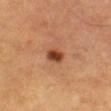follow-up — imaged on a skin check; not biopsied | subject — male, aged approximately 60 | automated lesion analysis — a lesion area of about 4.5 mm², a shape eccentricity near 0.6, and two-axis asymmetry of about 0.2; a border-irregularity rating of about 1.5/10, internal color variation of about 4 on a 0–10 scale, and radial color variation of about 1; an automated nevus-likeness rating near 100 out of 100 and a lesion-detection confidence of about 100/100 | imaging modality — ~15 mm tile from a whole-body skin photo | location — the left thigh.